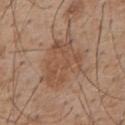The lesion was tiled from a total-body skin photograph and was not biopsied. The recorded lesion diameter is about 6.5 mm. A lesion tile, about 15 mm wide, cut from a 3D total-body photograph. The tile uses white-light illumination. The lesion is on the upper back. A male patient in their mid- to late 50s.